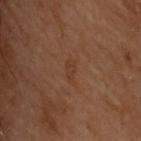Impression:
Captured during whole-body skin photography for melanoma surveillance; the lesion was not biopsied.
Image and clinical context:
A 15 mm crop from a total-body photograph taken for skin-cancer surveillance. A female subject, in their 60s. The lesion is located on the back. The recorded lesion diameter is about 2.5 mm. Imaged with cross-polarized lighting.